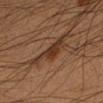No biopsy was performed on this lesion — it was imaged during a full skin examination and was not determined to be concerning. Located on the left forearm. About 5.5 mm across. A 15 mm crop from a total-body photograph taken for skin-cancer surveillance. A male patient in their mid-30s.